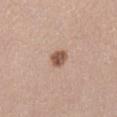biopsy status: total-body-photography surveillance lesion; no biopsy
image-analysis metrics: an outline eccentricity of about 0.55 (0 = round, 1 = elongated) and two-axis asymmetry of about 0.2; about 14 CIELAB-L* units darker than the surrounding skin and a normalized lesion–skin contrast near 9.5; a border-irregularity index near 1.5/10, a within-lesion color-variation index near 3/10, and a peripheral color-asymmetry measure near 1
lesion diameter: ~2.5 mm (longest diameter)
image: ~15 mm crop, total-body skin-cancer survey
patient: female, aged 58–62
illumination: white-light illumination
anatomic site: the leg